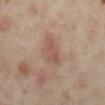Part of a total-body skin-imaging series; this lesion was reviewed on a skin check and was not flagged for biopsy.
The tile uses cross-polarized illumination.
Located on the right lower leg.
Approximately 3.5 mm at its widest.
A female subject aged approximately 35.
A 15 mm close-up extracted from a 3D total-body photography capture.
The total-body-photography lesion software estimated an area of roughly 5 mm² and a shape eccentricity near 0.9. And it measured an automated nevus-likeness rating near 40 out of 100 and a lesion-detection confidence of about 100/100.